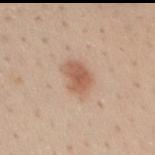biopsy_status: not biopsied; imaged during a skin examination
lesion_size:
  long_diameter_mm_approx: 3.5
patient:
  sex: male
  age_approx: 30
image:
  source: total-body photography crop
  field_of_view_mm: 15
site: mid back
lighting: white-light
automated_metrics:
  area_mm2_approx: 7.5
  eccentricity: 0.6
  shape_asymmetry: 0.2
  border_irregularity_0_10: 2.0
  nevus_likeness_0_100: 95
  lesion_detection_confidence_0_100: 100